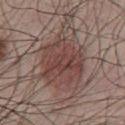Notes:
• workup — no biopsy performed (imaged during a skin exam)
• subject — male, aged 48–52
• body site — the chest
• image — ~15 mm crop, total-body skin-cancer survey